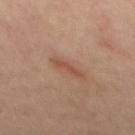Notes:
* follow-up: no biopsy performed (imaged during a skin exam)
* location: the mid back
* lesion size: about 3.5 mm
* image source: ~15 mm tile from a whole-body skin photo
* tile lighting: cross-polarized illumination
* patient: female, aged 43–47